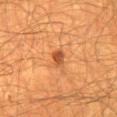The lesion was tiled from a total-body skin photograph and was not biopsied. A male subject, roughly 65 years of age. Located on the back. The tile uses cross-polarized illumination. A lesion tile, about 15 mm wide, cut from a 3D total-body photograph. The recorded lesion diameter is about 2.5 mm.